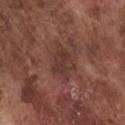Impression: No biopsy was performed on this lesion — it was imaged during a full skin examination and was not determined to be concerning. Context: From the chest. The patient is a male approximately 75 years of age. A roughly 15 mm field-of-view crop from a total-body skin photograph.